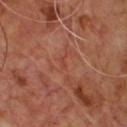Part of a total-body skin-imaging series; this lesion was reviewed on a skin check and was not flagged for biopsy. This is a cross-polarized tile. The patient is a male in their mid- to late 60s. The recorded lesion diameter is about 1.5 mm. A region of skin cropped from a whole-body photographic capture, roughly 15 mm wide.